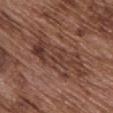Approximately 9.5 mm at its widest.
A male subject aged 73–77.
A roughly 15 mm field-of-view crop from a total-body skin photograph.
The lesion is located on the front of the torso.
Captured under white-light illumination.